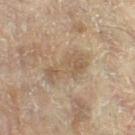No biopsy was performed on this lesion — it was imaged during a full skin examination and was not determined to be concerning.
Imaged with cross-polarized lighting.
The lesion is on the leg.
A close-up tile cropped from a whole-body skin photograph, about 15 mm across.
Longest diameter approximately 5 mm.
A female patient aged 78 to 82.